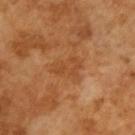Imaged during a routine full-body skin examination; the lesion was not biopsied and no histopathology is available.